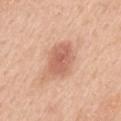Q: Was a biopsy performed?
A: total-body-photography surveillance lesion; no biopsy